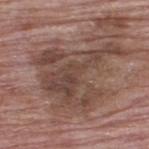Q: Was this lesion biopsied?
A: no biopsy performed (imaged during a skin exam)
Q: What are the patient's age and sex?
A: male, aged 63 to 67
Q: Automated lesion metrics?
A: an area of roughly 34 mm² and a shape-asymmetry score of about 0.55 (0 = symmetric); a lesion color around L≈44 a*≈17 b*≈23 in CIELAB and a lesion-to-skin contrast of about 7.5 (normalized; higher = more distinct); border irregularity of about 9 on a 0–10 scale, a within-lesion color-variation index near 5/10, and a peripheral color-asymmetry measure near 1.5
Q: Lesion size?
A: ~10 mm (longest diameter)
Q: What is the anatomic site?
A: the upper back
Q: How was the tile lit?
A: white-light illumination
Q: How was this image acquired?
A: total-body-photography crop, ~15 mm field of view